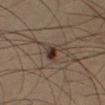Imaged during a routine full-body skin examination; the lesion was not biopsied and no histopathology is available.
A male patient aged approximately 35.
Measured at roughly 3 mm in maximum diameter.
The lesion is located on the right thigh.
An algorithmic analysis of the crop reported an average lesion color of about L≈30 a*≈12 b*≈21 (CIELAB), about 10 CIELAB-L* units darker than the surrounding skin, and a normalized lesion–skin contrast near 10. And it measured a within-lesion color-variation index near 7.5/10 and a peripheral color-asymmetry measure near 2.5. And it measured a classifier nevus-likeness of about 85/100 and lesion-presence confidence of about 100/100.
A 15 mm close-up tile from a total-body photography series done for melanoma screening.
This is a cross-polarized tile.